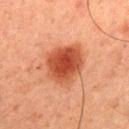Imaged during a routine full-body skin examination; the lesion was not biopsied and no histopathology is available. A male subject, aged approximately 60. Automated tile analysis of the lesion measured a mean CIELAB color near L≈39 a*≈27 b*≈31, about 12 CIELAB-L* units darker than the surrounding skin, and a lesion-to-skin contrast of about 10 (normalized; higher = more distinct). The analysis additionally found border irregularity of about 2 on a 0–10 scale. Located on the upper back. Cropped from a whole-body photographic skin survey; the tile spans about 15 mm. Imaged with cross-polarized lighting. The recorded lesion diameter is about 4.5 mm.